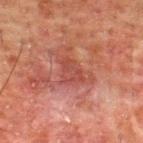The lesion was photographed on a routine skin check and not biopsied; there is no pathology result. The lesion is on the upper back. A male subject roughly 50 years of age. An algorithmic analysis of the crop reported an area of roughly 5.5 mm² and a shape eccentricity near 0.7. The recorded lesion diameter is about 3.5 mm. A 15 mm crop from a total-body photograph taken for skin-cancer surveillance.